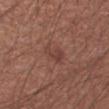Clinical impression: Part of a total-body skin-imaging series; this lesion was reviewed on a skin check and was not flagged for biopsy. Context: Located on the left forearm. Measured at roughly 3 mm in maximum diameter. Cropped from a whole-body photographic skin survey; the tile spans about 15 mm. A male subject, aged approximately 55. The tile uses white-light illumination.